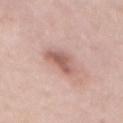Assessment: Captured during whole-body skin photography for melanoma surveillance; the lesion was not biopsied.